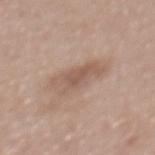biopsy status: imaged on a skin check; not biopsied
anatomic site: the mid back
image source: 15 mm crop, total-body photography
subject: female, aged 58 to 62
diameter: about 5 mm